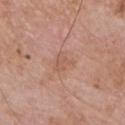This image is a 15 mm lesion crop taken from a total-body photograph. The subject is a male aged 58 to 62. Automated image analysis of the tile measured a lesion area of about 4 mm², an eccentricity of roughly 0.65, and two-axis asymmetry of about 0.4. It also reported a border-irregularity rating of about 4/10. The software also gave a classifier nevus-likeness of about 0/100 and a lesion-detection confidence of about 100/100. On the chest.